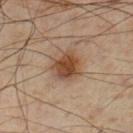Part of a total-body skin-imaging series; this lesion was reviewed on a skin check and was not flagged for biopsy.
A male subject, aged 53 to 57.
The tile uses cross-polarized illumination.
The lesion is on the left lower leg.
An algorithmic analysis of the crop reported a border-irregularity rating of about 2/10, a color-variation rating of about 4/10, and peripheral color asymmetry of about 1.5.
A 15 mm close-up tile from a total-body photography series done for melanoma screening.